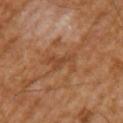Clinical impression:
The lesion was photographed on a routine skin check and not biopsied; there is no pathology result.
Context:
This is a cross-polarized tile. A male subject, in their mid- to late 60s. The lesion's longest dimension is about 4 mm. A 15 mm crop from a total-body photograph taken for skin-cancer surveillance. On the left upper arm. Automated image analysis of the tile measured an area of roughly 5.5 mm² and an outline eccentricity of about 0.85 (0 = round, 1 = elongated). The analysis additionally found a mean CIELAB color near L≈42 a*≈22 b*≈33, about 7 CIELAB-L* units darker than the surrounding skin, and a normalized lesion–skin contrast near 6. It also reported a nevus-likeness score of about 0/100.